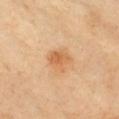follow-up — catalogued during a skin exam; not biopsied